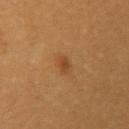The lesion was photographed on a routine skin check and not biopsied; there is no pathology result. A close-up tile cropped from a whole-body skin photograph, about 15 mm across. Automated image analysis of the tile measured a footprint of about 2.5 mm², an outline eccentricity of about 0.6 (0 = round, 1 = elongated), and a symmetry-axis asymmetry near 0.25. It also reported a mean CIELAB color near L≈41 a*≈20 b*≈36, a lesion–skin lightness drop of about 7, and a lesion-to-skin contrast of about 6 (normalized; higher = more distinct). The lesion is on the arm. A female patient aged around 30. This is a cross-polarized tile.